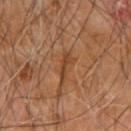| field | value |
|---|---|
| biopsy status | imaged on a skin check; not biopsied |
| tile lighting | cross-polarized illumination |
| size | ≈3 mm |
| subject | male, about 65 years old |
| acquisition | 15 mm crop, total-body photography |
| body site | the right upper arm |